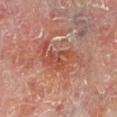Findings:
– follow-up — total-body-photography surveillance lesion; no biopsy
– TBP lesion metrics — a shape eccentricity near 0.85 and two-axis asymmetry of about 0.45; a border-irregularity index near 7/10, a within-lesion color-variation index near 5/10, and peripheral color asymmetry of about 1.5; a classifier nevus-likeness of about 0/100 and a lesion-detection confidence of about 95/100
– acquisition — ~15 mm tile from a whole-body skin photo
– lighting — cross-polarized illumination
– anatomic site — the left lower leg
– lesion diameter — about 6.5 mm
– subject — male, aged 58 to 62